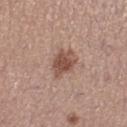<tbp_lesion>
<biopsy_status>not biopsied; imaged during a skin examination</biopsy_status>
<image>
  <source>total-body photography crop</source>
  <field_of_view_mm>15</field_of_view_mm>
</image>
<patient>
  <sex>female</sex>
  <age_approx>35</age_approx>
</patient>
<site>right lower leg</site>
<lighting>white-light</lighting>
<lesion_size>
  <long_diameter_mm_approx>4.0</long_diameter_mm_approx>
</lesion_size>
</tbp_lesion>